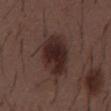notes = no biopsy performed (imaged during a skin exam)
anatomic site = the mid back
tile lighting = white-light illumination
lesion diameter = about 6 mm
imaging modality = ~15 mm crop, total-body skin-cancer survey
subject = male, in their 50s